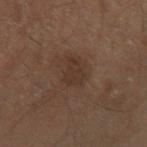| field | value |
|---|---|
| workup | catalogued during a skin exam; not biopsied |
| tile lighting | cross-polarized illumination |
| subject | male, about 60 years old |
| automated lesion analysis | an area of roughly 5.5 mm², a shape eccentricity near 0.7, and a symmetry-axis asymmetry near 0.4; an automated nevus-likeness rating near 5 out of 100 and lesion-presence confidence of about 100/100 |
| image source | 15 mm crop, total-body photography |
| site | the arm |